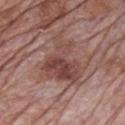{"biopsy_status": "not biopsied; imaged during a skin examination"}